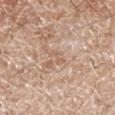Q: Was a biopsy performed?
A: imaged on a skin check; not biopsied
Q: What is the anatomic site?
A: the left lower leg
Q: How was the tile lit?
A: white-light
Q: What kind of image is this?
A: total-body-photography crop, ~15 mm field of view
Q: Patient demographics?
A: male, aged around 60
Q: Automated lesion metrics?
A: a border-irregularity rating of about 6/10, internal color variation of about 0 on a 0–10 scale, and a peripheral color-asymmetry measure near 0
Q: What is the lesion's diameter?
A: ~2.5 mm (longest diameter)